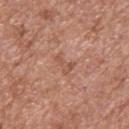notes: no biopsy performed (imaged during a skin exam)
site: the upper back
image-analysis metrics: a lesion area of about 3.5 mm², an outline eccentricity of about 0.85 (0 = round, 1 = elongated), and two-axis asymmetry of about 0.5; border irregularity of about 5.5 on a 0–10 scale, a color-variation rating of about 1.5/10, and a peripheral color-asymmetry measure near 1
patient: male, about 65 years old
image: 15 mm crop, total-body photography
lighting: white-light illumination